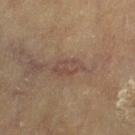Q: Is there a histopathology result?
A: no biopsy performed (imaged during a skin exam)
Q: Illumination type?
A: cross-polarized illumination
Q: Lesion location?
A: the left thigh
Q: What is the imaging modality?
A: ~15 mm crop, total-body skin-cancer survey
Q: What are the patient's age and sex?
A: female, aged 78 to 82